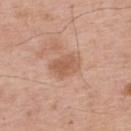Impression:
Recorded during total-body skin imaging; not selected for excision or biopsy.
Acquisition and patient details:
Approximately 3.5 mm at its widest. A male subject, aged approximately 65. Located on the upper back. This image is a 15 mm lesion crop taken from a total-body photograph. The total-body-photography lesion software estimated a shape eccentricity near 0.75 and a symmetry-axis asymmetry near 0.2. It also reported a border-irregularity index near 2/10 and internal color variation of about 2.5 on a 0–10 scale.